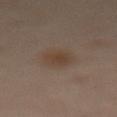| feature | finding |
|---|---|
| workup | imaged on a skin check; not biopsied |
| subject | female, aged 48 to 52 |
| site | the abdomen |
| acquisition | ~15 mm tile from a whole-body skin photo |